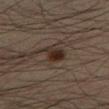Findings:
– notes: imaged on a skin check; not biopsied
– TBP lesion metrics: an average lesion color of about L≈25 a*≈13 b*≈20 (CIELAB), roughly 9 lightness units darker than nearby skin, and a lesion-to-skin contrast of about 10 (normalized; higher = more distinct)
– lighting: cross-polarized illumination
– lesion size: about 3 mm
– subject: male, in their mid- to late 30s
– image source: ~15 mm tile from a whole-body skin photo
– anatomic site: the right lower leg